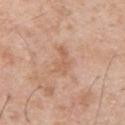notes = catalogued during a skin exam; not biopsied
illumination = white-light illumination
subject = male, in their mid- to late 50s
size = about 3.5 mm
imaging modality = ~15 mm crop, total-body skin-cancer survey
image-analysis metrics = a lesion color around L≈61 a*≈21 b*≈32 in CIELAB and roughly 7 lightness units darker than nearby skin; border irregularity of about 5 on a 0–10 scale, internal color variation of about 1.5 on a 0–10 scale, and radial color variation of about 0.5; an automated nevus-likeness rating near 0 out of 100 and a detector confidence of about 100 out of 100 that the crop contains a lesion
location = the upper back